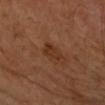follow-up = no biopsy performed (imaged during a skin exam) | body site = the left forearm | subject = female, about 60 years old | imaging modality = ~15 mm crop, total-body skin-cancer survey | lesion size = ≈3 mm | tile lighting = cross-polarized | automated lesion analysis = an area of roughly 4 mm² and an eccentricity of roughly 0.7; an average lesion color of about L≈33 a*≈23 b*≈32 (CIELAB), roughly 7 lightness units darker than nearby skin, and a normalized lesion–skin contrast near 7; a border-irregularity rating of about 3.5/10 and a peripheral color-asymmetry measure near 0.5; a classifier nevus-likeness of about 5/100 and lesion-presence confidence of about 100/100.